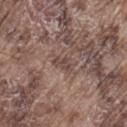<record>
  <biopsy_status>not biopsied; imaged during a skin examination</biopsy_status>
  <lesion_size>
    <long_diameter_mm_approx>2.5</long_diameter_mm_approx>
  </lesion_size>
  <automated_metrics>
    <area_mm2_approx>3.0</area_mm2_approx>
    <eccentricity>0.85</eccentricity>
    <shape_asymmetry>0.45</shape_asymmetry>
    <vs_skin_darker_L>8.0</vs_skin_darker_L>
    <vs_skin_contrast_norm>6.5</vs_skin_contrast_norm>
    <border_irregularity_0_10>5.0</border_irregularity_0_10>
    <color_variation_0_10>1.0</color_variation_0_10>
    <peripheral_color_asymmetry>0.5</peripheral_color_asymmetry>
  </automated_metrics>
  <site>left thigh</site>
  <patient>
    <sex>male</sex>
    <age_approx>75</age_approx>
  </patient>
  <image>
    <source>total-body photography crop</source>
    <field_of_view_mm>15</field_of_view_mm>
  </image>
</record>